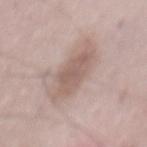- lesion diameter: ~6.5 mm (longest diameter)
- location: the back
- automated metrics: border irregularity of about 3.5 on a 0–10 scale and internal color variation of about 2.5 on a 0–10 scale
- subject: male, aged approximately 50
- acquisition: ~15 mm crop, total-body skin-cancer survey